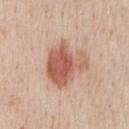biopsy_status: not biopsied; imaged during a skin examination
automated_metrics:
  area_mm2_approx: 18.0
  shape_asymmetry: 0.35
  color_variation_0_10: 6.5
  nevus_likeness_0_100: 95
  lesion_detection_confidence_0_100: 100
site: chest
lesion_size:
  long_diameter_mm_approx: 5.0
lighting: white-light
patient:
  sex: male
  age_approx: 60
image:
  source: total-body photography crop
  field_of_view_mm: 15